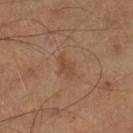Q: Was a biopsy performed?
A: imaged on a skin check; not biopsied
Q: How was this image acquired?
A: total-body-photography crop, ~15 mm field of view
Q: What is the anatomic site?
A: the left lower leg
Q: What is the lesion's diameter?
A: about 3 mm
Q: What did automated image analysis measure?
A: an automated nevus-likeness rating near 20 out of 100 and lesion-presence confidence of about 100/100
Q: What lighting was used for the tile?
A: cross-polarized illumination
Q: Who is the patient?
A: male, aged 43–47